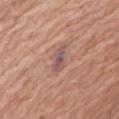| key | value |
|---|---|
| notes | no biopsy performed (imaged during a skin exam) |
| subject | female, about 75 years old |
| anatomic site | the mid back |
| diameter | about 3.5 mm |
| image source | ~15 mm crop, total-body skin-cancer survey |